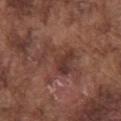follow-up: total-body-photography surveillance lesion; no biopsy
automated metrics: roughly 7 lightness units darker than nearby skin and a normalized border contrast of about 6.5; an automated nevus-likeness rating near 0 out of 100
diameter: ≈6.5 mm
subject: male, aged around 75
site: the chest
image source: 15 mm crop, total-body photography